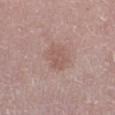notes = catalogued during a skin exam; not biopsied
illumination = white-light illumination
image = ~15 mm crop, total-body skin-cancer survey
patient = female, in their mid-60s
location = the leg
size = about 3 mm